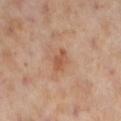biopsy status = imaged on a skin check; not biopsied
image = total-body-photography crop, ~15 mm field of view
lesion diameter = ~3 mm (longest diameter)
tile lighting = cross-polarized illumination
site = the left lower leg
patient = female, in their mid- to late 50s